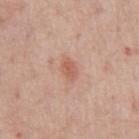  biopsy_status: not biopsied; imaged during a skin examination
  patient:
    sex: male
    age_approx: 60
  image:
    source: total-body photography crop
    field_of_view_mm: 15
  site: front of the torso
  automated_metrics:
    eccentricity: 0.75
    shape_asymmetry: 0.2
  lighting: white-light